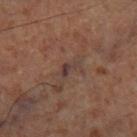follow-up = total-body-photography surveillance lesion; no biopsy | diameter = about 2.5 mm | illumination = cross-polarized illumination | image source = ~15 mm crop, total-body skin-cancer survey | automated lesion analysis = a shape eccentricity near 0.85; a lesion color around L≈38 a*≈17 b*≈20 in CIELAB and a normalized border contrast of about 8; a color-variation rating of about 0/10 and a peripheral color-asymmetry measure near 0; a classifier nevus-likeness of about 0/100 and a lesion-detection confidence of about 70/100 | site = the left lower leg.